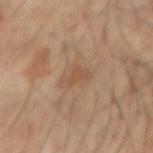Notes:
- workup: catalogued during a skin exam; not biopsied
- imaging modality: ~15 mm crop, total-body skin-cancer survey
- tile lighting: cross-polarized
- site: the right forearm
- size: ≈3.5 mm
- subject: male, approximately 55 years of age